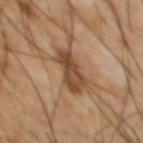Notes:
• follow-up — total-body-photography surveillance lesion; no biopsy
• tile lighting — cross-polarized illumination
• size — ~5 mm (longest diameter)
• acquisition — ~15 mm tile from a whole-body skin photo
• subject — male, about 65 years old
• body site — the chest